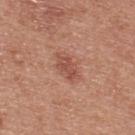Image and clinical context: On the upper back. A male patient, aged 28–32. This image is a 15 mm lesion crop taken from a total-body photograph. The tile uses white-light illumination. Automated tile analysis of the lesion measured a mean CIELAB color near L≈51 a*≈26 b*≈28, roughly 9 lightness units darker than nearby skin, and a normalized border contrast of about 6.5. The analysis additionally found a classifier nevus-likeness of about 20/100 and lesion-presence confidence of about 100/100. The recorded lesion diameter is about 3 mm.